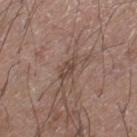  biopsy_status: not biopsied; imaged during a skin examination
  patient:
    sex: male
    age_approx: 20
  image:
    source: total-body photography crop
    field_of_view_mm: 15
  lesion_size:
    long_diameter_mm_approx: 3.5
  site: right lower leg